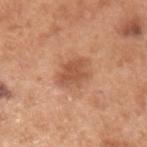Findings:
* lesion size: ≈4 mm
* tile lighting: white-light
* automated metrics: a mean CIELAB color near L≈54 a*≈25 b*≈34, roughly 10 lightness units darker than nearby skin, and a lesion-to-skin contrast of about 7 (normalized; higher = more distinct); a border-irregularity index near 3/10, a color-variation rating of about 2.5/10, and radial color variation of about 1
* anatomic site: the left upper arm
* patient: male, approximately 55 years of age
* image: ~15 mm tile from a whole-body skin photo